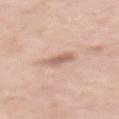No biopsy was performed on this lesion — it was imaged during a full skin examination and was not determined to be concerning. The lesion is located on the back. Longest diameter approximately 3 mm. An algorithmic analysis of the crop reported an outline eccentricity of about 0.8 (0 = round, 1 = elongated) and two-axis asymmetry of about 0.25. It also reported internal color variation of about 1.5 on a 0–10 scale. The analysis additionally found a nevus-likeness score of about 10/100. The patient is a female in their mid-50s. A region of skin cropped from a whole-body photographic capture, roughly 15 mm wide. The tile uses white-light illumination.